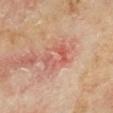The lesion was photographed on a routine skin check and not biopsied; there is no pathology result. A male patient, in their mid- to late 60s. Captured under cross-polarized illumination. A close-up tile cropped from a whole-body skin photograph, about 15 mm across. The lesion is on the left lower leg. About 4 mm across.